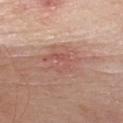Context: A female patient roughly 70 years of age. Longest diameter approximately 5 mm. A region of skin cropped from a whole-body photographic capture, roughly 15 mm wide. Imaged with white-light lighting. On the chest.